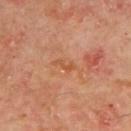location: the mid back
subject: male, aged 63 to 67
TBP lesion metrics: a lesion–skin lightness drop of about 6
tile lighting: cross-polarized illumination
image source: ~15 mm tile from a whole-body skin photo
diameter: about 2.5 mm